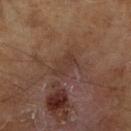Q: Was a biopsy performed?
A: catalogued during a skin exam; not biopsied
Q: Who is the patient?
A: male, approximately 65 years of age
Q: How was this image acquired?
A: total-body-photography crop, ~15 mm field of view
Q: Automated lesion metrics?
A: an area of roughly 6 mm², an outline eccentricity of about 0.8 (0 = round, 1 = elongated), and two-axis asymmetry of about 0.3; a mean CIELAB color near L≈35 a*≈17 b*≈24, about 5 CIELAB-L* units darker than the surrounding skin, and a lesion-to-skin contrast of about 5 (normalized; higher = more distinct)
Q: What lighting was used for the tile?
A: cross-polarized